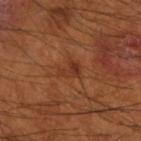Notes:
– workup · total-body-photography surveillance lesion; no biopsy
– lesion diameter · ~2.5 mm (longest diameter)
– anatomic site · the arm
– TBP lesion metrics · a lesion area of about 4 mm² and an outline eccentricity of about 0.7 (0 = round, 1 = elongated); a classifier nevus-likeness of about 0/100 and a lesion-detection confidence of about 100/100
– image · ~15 mm tile from a whole-body skin photo
– subject · male, aged 53–57
– lighting · cross-polarized illumination